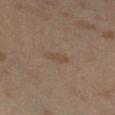notes = total-body-photography surveillance lesion; no biopsy | patient = female, approximately 40 years of age | lesion diameter = about 2.5 mm | imaging modality = ~15 mm crop, total-body skin-cancer survey | location = the left leg.